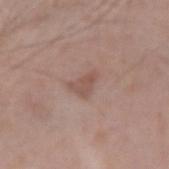follow-up = imaged on a skin check; not biopsied | patient = male, aged 58–62 | site = the right upper arm | acquisition = total-body-photography crop, ~15 mm field of view | automated metrics = an eccentricity of roughly 0.7; border irregularity of about 5 on a 0–10 scale, internal color variation of about 1.5 on a 0–10 scale, and radial color variation of about 0.5 | illumination = white-light.